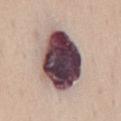<tbp_lesion>
<biopsy_status>not biopsied; imaged during a skin examination</biopsy_status>
<patient>
  <sex>male</sex>
  <age_approx>75</age_approx>
</patient>
<image>
  <source>total-body photography crop</source>
  <field_of_view_mm>15</field_of_view_mm>
</image>
<site>lower back</site>
</tbp_lesion>